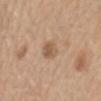Imaged during a routine full-body skin examination; the lesion was not biopsied and no histopathology is available. The lesion-visualizer software estimated a shape eccentricity near 0.65. And it measured border irregularity of about 1.5 on a 0–10 scale and a color-variation rating of about 3/10. It also reported a classifier nevus-likeness of about 25/100. Cropped from a total-body skin-imaging series; the visible field is about 15 mm. From the front of the torso. Measured at roughly 3 mm in maximum diameter. The subject is a female aged 68–72.